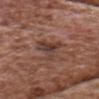Clinical impression: Imaged during a routine full-body skin examination; the lesion was not biopsied and no histopathology is available. Background: The lesion is on the head or neck. A male subject, in their mid-70s. A region of skin cropped from a whole-body photographic capture, roughly 15 mm wide.